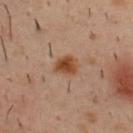No biopsy was performed on this lesion — it was imaged during a full skin examination and was not determined to be concerning. Measured at roughly 3 mm in maximum diameter. Automated image analysis of the tile measured a footprint of about 5.5 mm², an outline eccentricity of about 0.6 (0 = round, 1 = elongated), and a symmetry-axis asymmetry near 0.25. And it measured roughly 11 lightness units darker than nearby skin and a normalized lesion–skin contrast near 10. And it measured a border-irregularity index near 2/10 and internal color variation of about 4 on a 0–10 scale. And it measured an automated nevus-likeness rating near 100 out of 100. A lesion tile, about 15 mm wide, cut from a 3D total-body photograph. The tile uses cross-polarized illumination. The patient is a male approximately 40 years of age. The lesion is on the upper back.